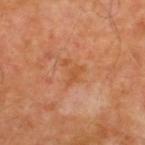<tbp_lesion>
<biopsy_status>not biopsied; imaged during a skin examination</biopsy_status>
<lesion_size>
  <long_diameter_mm_approx>3.0</long_diameter_mm_approx>
</lesion_size>
<patient>
  <sex>male</sex>
  <age_approx>55</age_approx>
</patient>
<site>upper back</site>
<automated_metrics>
  <area_mm2_approx>4.0</area_mm2_approx>
  <eccentricity>0.65</eccentricity>
  <shape_asymmetry>0.55</shape_asymmetry>
  <border_irregularity_0_10>6.0</border_irregularity_0_10>
  <color_variation_0_10>1.0</color_variation_0_10>
  <peripheral_color_asymmetry>0.0</peripheral_color_asymmetry>
  <nevus_likeness_0_100>0</nevus_likeness_0_100>
</automated_metrics>
<image>
  <source>total-body photography crop</source>
  <field_of_view_mm>15</field_of_view_mm>
</image>
</tbp_lesion>